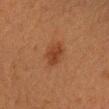Recorded during total-body skin imaging; not selected for excision or biopsy. Imaged with cross-polarized lighting. Longest diameter approximately 3 mm. A female subject in their 40s. Automated tile analysis of the lesion measured a border-irregularity rating of about 2/10, a color-variation rating of about 2/10, and a peripheral color-asymmetry measure near 0.5. On the arm. Cropped from a whole-body photographic skin survey; the tile spans about 15 mm.